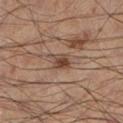Part of a total-body skin-imaging series; this lesion was reviewed on a skin check and was not flagged for biopsy. This image is a 15 mm lesion crop taken from a total-body photograph. The recorded lesion diameter is about 2.5 mm. The tile uses cross-polarized illumination. A male subject, about 55 years old. The total-body-photography lesion software estimated a border-irregularity index near 3.5/10, a within-lesion color-variation index near 3/10, and radial color variation of about 1. It also reported a classifier nevus-likeness of about 80/100 and a detector confidence of about 100 out of 100 that the crop contains a lesion. Located on the left lower leg.